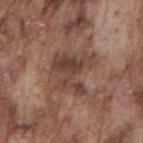The lesion was photographed on a routine skin check and not biopsied; there is no pathology result.
Measured at roughly 5 mm in maximum diameter.
Cropped from a whole-body photographic skin survey; the tile spans about 15 mm.
On the mid back.
The patient is a male approximately 75 years of age.
This is a white-light tile.